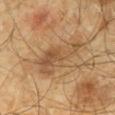This lesion was catalogued during total-body skin photography and was not selected for biopsy. Captured under cross-polarized illumination. A lesion tile, about 15 mm wide, cut from a 3D total-body photograph. A male patient in their mid-60s. The lesion is located on the mid back. The total-body-photography lesion software estimated a footprint of about 14 mm², an outline eccentricity of about 0.9 (0 = round, 1 = elongated), and two-axis asymmetry of about 0.45. It also reported a mean CIELAB color near L≈49 a*≈17 b*≈34, roughly 9 lightness units darker than nearby skin, and a lesion-to-skin contrast of about 6.5 (normalized; higher = more distinct). The analysis additionally found a border-irregularity rating of about 6/10, a within-lesion color-variation index near 4/10, and peripheral color asymmetry of about 1. The software also gave a classifier nevus-likeness of about 0/100 and a detector confidence of about 100 out of 100 that the crop contains a lesion. Measured at roughly 6.5 mm in maximum diameter.